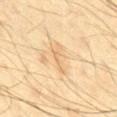Captured during whole-body skin photography for melanoma surveillance; the lesion was not biopsied.
Imaged with cross-polarized lighting.
Located on the mid back.
A male subject, in their mid- to late 70s.
About 4 mm across.
A 15 mm close-up extracted from a 3D total-body photography capture.